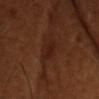Q: Was this lesion biopsied?
A: catalogued during a skin exam; not biopsied
Q: What is the anatomic site?
A: the chest
Q: Illumination type?
A: cross-polarized illumination
Q: What are the patient's age and sex?
A: female, aged approximately 55
Q: What kind of image is this?
A: total-body-photography crop, ~15 mm field of view
Q: Lesion size?
A: about 4 mm